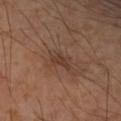Image and clinical context: Cropped from a total-body skin-imaging series; the visible field is about 15 mm. Captured under cross-polarized illumination. Located on the arm. The recorded lesion diameter is about 3 mm.